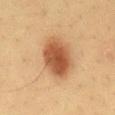Assessment: No biopsy was performed on this lesion — it was imaged during a full skin examination and was not determined to be concerning. Image and clinical context: The subject is a male in their mid- to late 30s. A 15 mm crop from a total-body photograph taken for skin-cancer surveillance. From the back. Measured at roughly 6 mm in maximum diameter.